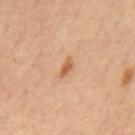Q: Is there a histopathology result?
A: catalogued during a skin exam; not biopsied
Q: Lesion location?
A: the mid back
Q: What is the imaging modality?
A: 15 mm crop, total-body photography
Q: What is the lesion's diameter?
A: ≈2.5 mm
Q: Who is the patient?
A: male, about 60 years old
Q: What did automated image analysis measure?
A: a border-irregularity rating of about 3/10, internal color variation of about 0.5 on a 0–10 scale, and radial color variation of about 0; lesion-presence confidence of about 100/100
Q: What lighting was used for the tile?
A: cross-polarized illumination